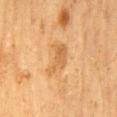{
  "biopsy_status": "not biopsied; imaged during a skin examination",
  "lighting": "cross-polarized",
  "patient": {
    "sex": "male",
    "age_approx": 60
  },
  "lesion_size": {
    "long_diameter_mm_approx": 4.5
  },
  "site": "mid back",
  "image": {
    "source": "total-body photography crop",
    "field_of_view_mm": 15
  }
}